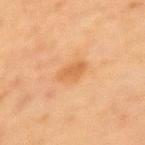Q: Is there a histopathology result?
A: catalogued during a skin exam; not biopsied
Q: What is the imaging modality?
A: ~15 mm crop, total-body skin-cancer survey
Q: What lighting was used for the tile?
A: cross-polarized illumination
Q: What did automated image analysis measure?
A: a footprint of about 5 mm² and a shape eccentricity near 0.75; a lesion–skin lightness drop of about 7; an automated nevus-likeness rating near 10 out of 100 and a lesion-detection confidence of about 100/100
Q: Where on the body is the lesion?
A: the left upper arm
Q: Lesion size?
A: about 3 mm
Q: Who is the patient?
A: female, approximately 55 years of age